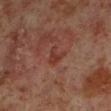Clinical impression:
No biopsy was performed on this lesion — it was imaged during a full skin examination and was not determined to be concerning.